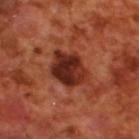Impression: Captured during whole-body skin photography for melanoma surveillance; the lesion was not biopsied. Clinical summary: The total-body-photography lesion software estimated a footprint of about 14 mm² and two-axis asymmetry of about 0.25. It also reported a border-irregularity index near 2.5/10, a within-lesion color-variation index near 7/10, and a peripheral color-asymmetry measure near 2.5. The software also gave an automated nevus-likeness rating near 75 out of 100 and a lesion-detection confidence of about 100/100. On the upper back. A roughly 15 mm field-of-view crop from a total-body skin photograph. The subject is a male in their 70s. Measured at roughly 5 mm in maximum diameter.